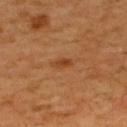  biopsy_status: not biopsied; imaged during a skin examination
  site: upper back
  automated_metrics:
    shape_asymmetry: 0.3
    cielab_L: 42
    cielab_a: 24
    cielab_b: 38
    vs_skin_contrast_norm: 6.5
    border_irregularity_0_10: 3.0
    nevus_likeness_0_100: 75
    lesion_detection_confidence_0_100: 100
  lesion_size:
    long_diameter_mm_approx: 2.5
  lighting: cross-polarized
  patient:
    sex: male
    age_approx: 60
  image:
    source: total-body photography crop
    field_of_view_mm: 15